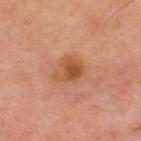Assessment:
Part of a total-body skin-imaging series; this lesion was reviewed on a skin check and was not flagged for biopsy.
Clinical summary:
The tile uses cross-polarized illumination. The patient is a male in their 50s. The total-body-photography lesion software estimated an area of roughly 6.5 mm², an outline eccentricity of about 0.55 (0 = round, 1 = elongated), and a shape-asymmetry score of about 0.35 (0 = symmetric). And it measured a border-irregularity index near 3.5/10 and a peripheral color-asymmetry measure near 1.5. It also reported an automated nevus-likeness rating near 55 out of 100 and a lesion-detection confidence of about 100/100. Measured at roughly 3.5 mm in maximum diameter. A 15 mm close-up extracted from a 3D total-body photography capture. The lesion is on the back.